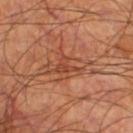The lesion was photographed on a routine skin check and not biopsied; there is no pathology result.
A 15 mm close-up extracted from a 3D total-body photography capture.
This is a cross-polarized tile.
From the right thigh.
A male patient, aged 58–62.
Longest diameter approximately 4 mm.